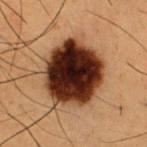The lesion was photographed on a routine skin check and not biopsied; there is no pathology result.